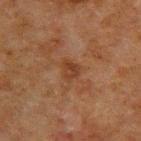  biopsy_status: not biopsied; imaged during a skin examination
  automated_metrics:
    cielab_L: 32
    cielab_a: 19
    cielab_b: 28
    vs_skin_darker_L: 6.0
    vs_skin_contrast_norm: 6.5
    border_irregularity_0_10: 3.5
    peripheral_color_asymmetry: 1.0
    nevus_likeness_0_100: 0
    lesion_detection_confidence_0_100: 100
  patient:
    sex: male
    age_approx: 50
  image:
    source: total-body photography crop
    field_of_view_mm: 15
  site: upper back
  lesion_size:
    long_diameter_mm_approx: 2.5
  lighting: cross-polarized